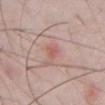Impression:
No biopsy was performed on this lesion — it was imaged during a full skin examination and was not determined to be concerning.
Image and clinical context:
A roughly 15 mm field-of-view crop from a total-body skin photograph. This is a white-light tile. The lesion is on the chest. A male subject about 65 years old.